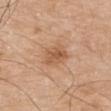Q: How was the tile lit?
A: white-light
Q: What are the patient's age and sex?
A: male, roughly 80 years of age
Q: What is the imaging modality?
A: 15 mm crop, total-body photography
Q: What is the lesion's diameter?
A: about 3.5 mm
Q: Lesion location?
A: the left upper arm